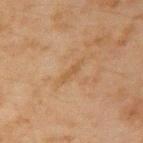  biopsy_status: not biopsied; imaged during a skin examination
  lighting: cross-polarized
  automated_metrics:
    cielab_L: 44
    cielab_a: 16
    cielab_b: 32
    vs_skin_darker_L: 5.0
    vs_skin_contrast_norm: 5.0
    nevus_likeness_0_100: 0
    lesion_detection_confidence_0_100: 100
  lesion_size:
    long_diameter_mm_approx: 3.0
  site: right upper arm
  patient:
    sex: male
    age_approx: 45
  image:
    source: total-body photography crop
    field_of_view_mm: 15